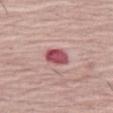Imaged during a routine full-body skin examination; the lesion was not biopsied and no histopathology is available. Imaged with white-light lighting. The lesion is located on the left thigh. Cropped from a total-body skin-imaging series; the visible field is about 15 mm. The total-body-photography lesion software estimated an area of roughly 5.5 mm², an eccentricity of roughly 0.6, and a symmetry-axis asymmetry near 0.2. The subject is a female aged 63 to 67.